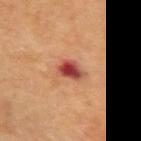follow-up: total-body-photography surveillance lesion; no biopsy
patient: male, in their mid- to late 60s
anatomic site: the left upper arm
size: ~3 mm (longest diameter)
automated lesion analysis: an average lesion color of about L≈44 a*≈30 b*≈28 (CIELAB), about 14 CIELAB-L* units darker than the surrounding skin, and a normalized border contrast of about 11.5; a border-irregularity rating of about 2.5/10 and a peripheral color-asymmetry measure near 2.5
illumination: cross-polarized
image source: total-body-photography crop, ~15 mm field of view